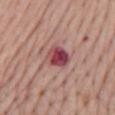This lesion was catalogued during total-body skin photography and was not selected for biopsy. The lesion-visualizer software estimated a lesion color around L≈46 a*≈30 b*≈19 in CIELAB and a normalized border contrast of about 11. It also reported a color-variation rating of about 9/10 and radial color variation of about 2.5. The recorded lesion diameter is about 3 mm. From the mid back. A female subject, roughly 65 years of age. A region of skin cropped from a whole-body photographic capture, roughly 15 mm wide.